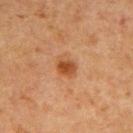Assessment: This lesion was catalogued during total-body skin photography and was not selected for biopsy. Acquisition and patient details: Automated tile analysis of the lesion measured a footprint of about 5.5 mm², an eccentricity of roughly 0.65, and a symmetry-axis asymmetry near 0.15. The software also gave a lesion–skin lightness drop of about 9. And it measured border irregularity of about 1.5 on a 0–10 scale, a within-lesion color-variation index near 5.5/10, and peripheral color asymmetry of about 1.5. On the mid back. About 3 mm across. A male patient aged around 65. A close-up tile cropped from a whole-body skin photograph, about 15 mm across. Captured under cross-polarized illumination.